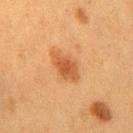Assessment:
This lesion was catalogued during total-body skin photography and was not selected for biopsy.
Background:
Located on the chest. Cropped from a whole-body photographic skin survey; the tile spans about 15 mm. Imaged with cross-polarized lighting. The subject is a female roughly 50 years of age. The recorded lesion diameter is about 4.5 mm.